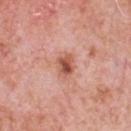Case summary:
• biopsy status — total-body-photography surveillance lesion; no biopsy